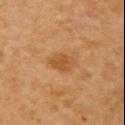{"biopsy_status": "not biopsied; imaged during a skin examination", "site": "chest", "lighting": "cross-polarized", "image": {"source": "total-body photography crop", "field_of_view_mm": 15}, "patient": {"sex": "female", "age_approx": 55}, "lesion_size": {"long_diameter_mm_approx": 3.0}}